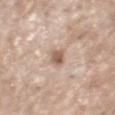| key | value |
|---|---|
| follow-up | catalogued during a skin exam; not biopsied |
| automated lesion analysis | a footprint of about 4 mm², an outline eccentricity of about 0.75 (0 = round, 1 = elongated), and two-axis asymmetry of about 0.25; a normalized border contrast of about 8; an automated nevus-likeness rating near 15 out of 100 |
| image source | total-body-photography crop, ~15 mm field of view |
| illumination | white-light illumination |
| anatomic site | the head or neck |
| lesion size | ~2.5 mm (longest diameter) |
| patient | male, aged 68–72 |